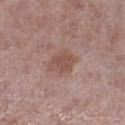<tbp_lesion>
<biopsy_status>not biopsied; imaged during a skin examination</biopsy_status>
<lesion_size>
  <long_diameter_mm_approx>3.5</long_diameter_mm_approx>
</lesion_size>
<patient>
  <sex>male</sex>
  <age_approx>70</age_approx>
</patient>
<lighting>white-light</lighting>
<image>
  <source>total-body photography crop</source>
  <field_of_view_mm>15</field_of_view_mm>
</image>
<site>right lower leg</site>
</tbp_lesion>